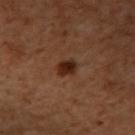Imaged during a routine full-body skin examination; the lesion was not biopsied and no histopathology is available.
A lesion tile, about 15 mm wide, cut from a 3D total-body photograph.
Located on the right upper arm.
The recorded lesion diameter is about 2.5 mm.
A male patient in their mid- to late 50s.
The total-body-photography lesion software estimated an area of roughly 4.5 mm², a shape eccentricity near 0.6, and a symmetry-axis asymmetry near 0.2. The software also gave an automated nevus-likeness rating near 100 out of 100 and a detector confidence of about 100 out of 100 that the crop contains a lesion.